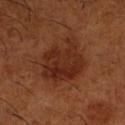The lesion was tiled from a total-body skin photograph and was not biopsied. The lesion-visualizer software estimated an area of roughly 20 mm², an outline eccentricity of about 0.45 (0 = round, 1 = elongated), and two-axis asymmetry of about 0.3. Cropped from a whole-body photographic skin survey; the tile spans about 15 mm. Approximately 6 mm at its widest. The patient is a male about 65 years old. From the right lower leg. Imaged with cross-polarized lighting.